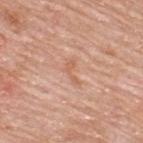  biopsy_status: not biopsied; imaged during a skin examination
  lesion_size:
    long_diameter_mm_approx: 3.0
  automated_metrics:
    nevus_likeness_0_100: 0
    lesion_detection_confidence_0_100: 100
  image:
    source: total-body photography crop
    field_of_view_mm: 15
  site: back
  lighting: white-light
  patient:
    sex: male
    age_approx: 80